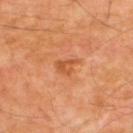Recorded during total-body skin imaging; not selected for excision or biopsy.
The lesion is on the upper back.
Longest diameter approximately 2.5 mm.
The tile uses cross-polarized illumination.
A male patient aged 53 to 57.
A 15 mm close-up extracted from a 3D total-body photography capture.